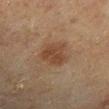biopsy_status: not biopsied; imaged during a skin examination
patient:
  sex: male
  age_approx: 65
site: leg
lesion_size:
  long_diameter_mm_approx: 4.0
automated_metrics:
  area_mm2_approx: 10.0
  eccentricity: 0.7
  cielab_L: 34
  cielab_a: 15
  cielab_b: 26
  vs_skin_contrast_norm: 7.0
  border_irregularity_0_10: 3.0
  color_variation_0_10: 2.5
  peripheral_color_asymmetry: 1.0
  nevus_likeness_0_100: 70
  lesion_detection_confidence_0_100: 100
image:
  source: total-body photography crop
  field_of_view_mm: 15